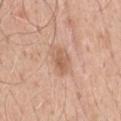Captured during whole-body skin photography for melanoma surveillance; the lesion was not biopsied. A male patient aged around 50. The recorded lesion diameter is about 4.5 mm. Located on the chest. Imaged with white-light lighting. Automated image analysis of the tile measured a lesion color around L≈63 a*≈19 b*≈31 in CIELAB, about 8 CIELAB-L* units darker than the surrounding skin, and a lesion-to-skin contrast of about 5 (normalized; higher = more distinct). And it measured a border-irregularity rating of about 2.5/10, a color-variation rating of about 4/10, and a peripheral color-asymmetry measure near 1. It also reported an automated nevus-likeness rating near 10 out of 100 and a detector confidence of about 100 out of 100 that the crop contains a lesion. A lesion tile, about 15 mm wide, cut from a 3D total-body photograph.